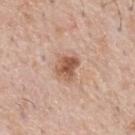biopsy status: no biopsy performed (imaged during a skin exam)
automated metrics: an area of roughly 7 mm², an eccentricity of roughly 0.5, and a shape-asymmetry score of about 0.25 (0 = symmetric); a lesion color around L≈57 a*≈21 b*≈30 in CIELAB and roughly 12 lightness units darker than nearby skin; internal color variation of about 5.5 on a 0–10 scale and a peripheral color-asymmetry measure near 2; a lesion-detection confidence of about 100/100
site: the mid back
imaging modality: total-body-photography crop, ~15 mm field of view
subject: male, aged 53–57
size: about 3.5 mm
illumination: white-light illumination